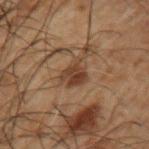biopsy status = total-body-photography surveillance lesion; no biopsy | image source = total-body-photography crop, ~15 mm field of view | patient = male, roughly 50 years of age | location = the left upper arm | size = ~4 mm (longest diameter) | illumination = cross-polarized illumination.